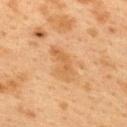notes — imaged on a skin check; not biopsied | automated metrics — a nevus-likeness score of about 0/100 and a lesion-detection confidence of about 100/100 | image — ~15 mm crop, total-body skin-cancer survey | body site — the upper back | subject — female, about 40 years old.